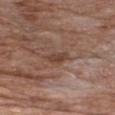No biopsy was performed on this lesion — it was imaged during a full skin examination and was not determined to be concerning.
Cropped from a whole-body photographic skin survey; the tile spans about 15 mm.
On the chest.
Imaged with white-light lighting.
About 3 mm across.
The total-body-photography lesion software estimated a lesion area of about 3.5 mm² and a shape eccentricity near 0.85. It also reported an automated nevus-likeness rating near 0 out of 100.
A female subject, aged around 75.